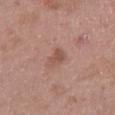Findings:
* tile lighting: white-light
* lesion size: ≈3 mm
* body site: the left thigh
* imaging modality: 15 mm crop, total-body photography
* subject: male, aged 48–52